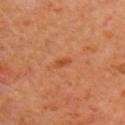biopsy status=total-body-photography surveillance lesion; no biopsy | subject=female, aged around 40 | tile lighting=cross-polarized illumination | image source=~15 mm tile from a whole-body skin photo | site=the arm.